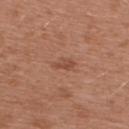{
  "biopsy_status": "not biopsied; imaged during a skin examination",
  "patient": {
    "sex": "female",
    "age_approx": 40
  },
  "image": {
    "source": "total-body photography crop",
    "field_of_view_mm": 15
  },
  "site": "upper back",
  "lesion_size": {
    "long_diameter_mm_approx": 2.5
  }
}